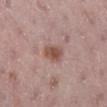<record>
  <lighting>white-light</lighting>
  <lesion_size>
    <long_diameter_mm_approx>3.0</long_diameter_mm_approx>
  </lesion_size>
  <image>
    <source>total-body photography crop</source>
    <field_of_view_mm>15</field_of_view_mm>
  </image>
  <automated_metrics>
    <cielab_L>51</cielab_L>
    <cielab_a>20</cielab_a>
    <cielab_b>26</cielab_b>
    <vs_skin_darker_L>11.0</vs_skin_darker_L>
    <vs_skin_contrast_norm>8.5</vs_skin_contrast_norm>
  </automated_metrics>
  <site>right lower leg</site>
  <patient>
    <sex>female</sex>
    <age_approx>45</age_approx>
  </patient>
</record>